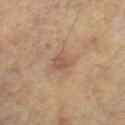Imaged during a routine full-body skin examination; the lesion was not biopsied and no histopathology is available. The lesion is located on the leg. The patient is a male aged approximately 60. This image is a 15 mm lesion crop taken from a total-body photograph.